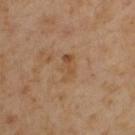Findings:
* biopsy status · no biopsy performed (imaged during a skin exam)
* TBP lesion metrics · a nevus-likeness score of about 0/100 and lesion-presence confidence of about 100/100
* site · the left upper arm
* subject · male, roughly 55 years of age
* acquisition · 15 mm crop, total-body photography